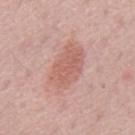{"biopsy_status": "not biopsied; imaged during a skin examination", "patient": {"sex": "male", "age_approx": 65}, "lighting": "white-light", "site": "back", "image": {"source": "total-body photography crop", "field_of_view_mm": 15}}